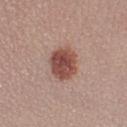Acquisition and patient details:
A region of skin cropped from a whole-body photographic capture, roughly 15 mm wide. A female patient, in their mid-20s. Approximately 4.5 mm at its widest. Captured under white-light illumination. The lesion is on the right lower leg.